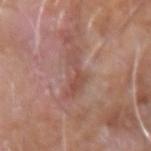  biopsy_status: not biopsied; imaged during a skin examination
  image:
    source: total-body photography crop
    field_of_view_mm: 15
  site: arm
  lighting: white-light
  lesion_size:
    long_diameter_mm_approx: 4.5
  patient:
    sex: male
    age_approx: 75
  automated_metrics:
    area_mm2_approx: 6.0
    eccentricity: 0.9
    shape_asymmetry: 0.65
    cielab_L: 50
    cielab_a: 22
    cielab_b: 26
    vs_skin_darker_L: 8.0
    vs_skin_contrast_norm: 6.0
    nevus_likeness_0_100: 0
    lesion_detection_confidence_0_100: 85